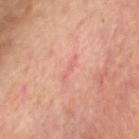- biopsy status — imaged on a skin check; not biopsied
- subject — male, aged approximately 55
- size — about 3 mm
- image source — total-body-photography crop, ~15 mm field of view
- site — the head or neck
- lighting — cross-polarized illumination